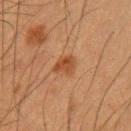The lesion was photographed on a routine skin check and not biopsied; there is no pathology result.
The patient is a male about 50 years old.
The lesion is located on the left upper arm.
Captured under cross-polarized illumination.
Approximately 2.5 mm at its widest.
A 15 mm close-up tile from a total-body photography series done for melanoma screening.